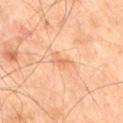Imaged during a routine full-body skin examination; the lesion was not biopsied and no histopathology is available. A male subject, aged 68 to 72. Captured under cross-polarized illumination. Cropped from a total-body skin-imaging series; the visible field is about 15 mm. On the leg. Measured at roughly 2.5 mm in maximum diameter. The lesion-visualizer software estimated a lesion area of about 3.5 mm² and a symmetry-axis asymmetry near 0.3. The software also gave a mean CIELAB color near L≈68 a*≈23 b*≈38 and roughly 8 lightness units darker than nearby skin. It also reported a border-irregularity index near 3.5/10, internal color variation of about 2 on a 0–10 scale, and peripheral color asymmetry of about 1. The software also gave a detector confidence of about 100 out of 100 that the crop contains a lesion.